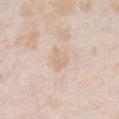Impression:
The lesion was photographed on a routine skin check and not biopsied; there is no pathology result.
Image and clinical context:
A lesion tile, about 15 mm wide, cut from a 3D total-body photograph. A female patient, about 25 years old. The lesion is located on the chest.